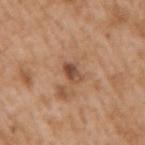Assessment:
This lesion was catalogued during total-body skin photography and was not selected for biopsy.
Context:
The tile uses white-light illumination. A lesion tile, about 15 mm wide, cut from a 3D total-body photograph. From the right upper arm. The patient is a male in their mid- to late 60s.